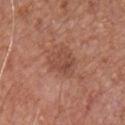{"biopsy_status": "not biopsied; imaged during a skin examination", "lesion_size": {"long_diameter_mm_approx": 4.0}, "image": {"source": "total-body photography crop", "field_of_view_mm": 15}, "lighting": "white-light", "patient": {"sex": "male", "age_approx": 65}, "site": "front of the torso"}